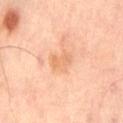Imaged during a routine full-body skin examination; the lesion was not biopsied and no histopathology is available.
On the left thigh.
Longest diameter approximately 3 mm.
A 15 mm close-up extracted from a 3D total-body photography capture.
A male patient aged approximately 65.